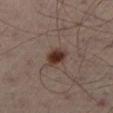notes — total-body-photography surveillance lesion; no biopsy | size — ~3.5 mm (longest diameter) | lighting — cross-polarized | subject — male, aged 48–52 | image — total-body-photography crop, ~15 mm field of view | location — the left leg | image-analysis metrics — a footprint of about 5.5 mm² and two-axis asymmetry of about 0.2; an average lesion color of about L≈33 a*≈17 b*≈23 (CIELAB), a lesion–skin lightness drop of about 12, and a lesion-to-skin contrast of about 11.5 (normalized; higher = more distinct); peripheral color asymmetry of about 1.5; an automated nevus-likeness rating near 100 out of 100 and a detector confidence of about 100 out of 100 that the crop contains a lesion.